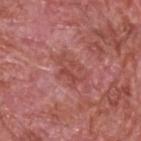notes: total-body-photography surveillance lesion; no biopsy
location: the head or neck
size: about 3.5 mm
image-analysis metrics: a lesion color around L≈48 a*≈29 b*≈26 in CIELAB, roughly 7 lightness units darker than nearby skin, and a normalized border contrast of about 5.5; border irregularity of about 6 on a 0–10 scale and a peripheral color-asymmetry measure near 1.5
image source: total-body-photography crop, ~15 mm field of view
subject: male, aged around 60